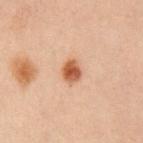biopsy status: imaged on a skin check; not biopsied
location: the left arm
size: ~2.5 mm (longest diameter)
patient: female, roughly 30 years of age
tile lighting: cross-polarized
image: 15 mm crop, total-body photography